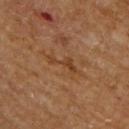notes = catalogued during a skin exam; not biopsied
subject = male, in their mid-60s
lighting = cross-polarized
anatomic site = the back
image = ~15 mm crop, total-body skin-cancer survey
size = about 4.5 mm